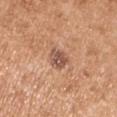notes = total-body-photography surveillance lesion; no biopsy
subject = male, about 55 years old
lesion size = about 3 mm
image = ~15 mm tile from a whole-body skin photo
location = the right upper arm